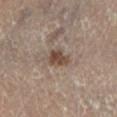follow-up: catalogued during a skin exam; not biopsied | location: the leg | diameter: about 2.5 mm | subject: female, approximately 60 years of age | imaging modality: 15 mm crop, total-body photography.